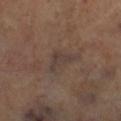This lesion was catalogued during total-body skin photography and was not selected for biopsy.
The lesion-visualizer software estimated a shape eccentricity near 0.85 and a shape-asymmetry score of about 0.65 (0 = symmetric). It also reported a mean CIELAB color near L≈38 a*≈13 b*≈20, about 6 CIELAB-L* units darker than the surrounding skin, and a normalized border contrast of about 6.5. The analysis additionally found a border-irregularity rating of about 7/10. The analysis additionally found a nevus-likeness score of about 0/100 and a lesion-detection confidence of about 60/100.
This image is a 15 mm lesion crop taken from a total-body photograph.
From the right lower leg.